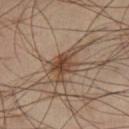{
  "site": "right lower leg",
  "lesion_size": {
    "long_diameter_mm_approx": 5.0
  },
  "image": {
    "source": "total-body photography crop",
    "field_of_view_mm": 15
  },
  "patient": {
    "sex": "male",
    "age_approx": 40
  },
  "lighting": "cross-polarized"
}